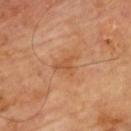Assessment: The lesion was photographed on a routine skin check and not biopsied; there is no pathology result. Context: The tile uses cross-polarized illumination. The patient is a male aged around 60. Approximately 2.5 mm at its widest. Automated image analysis of the tile measured a border-irregularity index near 5.5/10 and peripheral color asymmetry of about 0.5. A 15 mm close-up extracted from a 3D total-body photography capture. The lesion is located on the upper back.